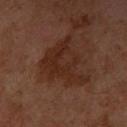Assessment: The lesion was tiled from a total-body skin photograph and was not biopsied. Clinical summary: A 15 mm close-up extracted from a 3D total-body photography capture. This is a cross-polarized tile. A female subject about 60 years old. From the arm. Automated image analysis of the tile measured a lesion–skin lightness drop of about 6 and a normalized border contrast of about 7.5. The analysis additionally found a border-irregularity rating of about 6.5/10, a within-lesion color-variation index near 3/10, and a peripheral color-asymmetry measure near 1. The analysis additionally found an automated nevus-likeness rating near 0 out of 100.